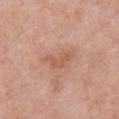The lesion was photographed on a routine skin check and not biopsied; there is no pathology result.
Cropped from a total-body skin-imaging series; the visible field is about 15 mm.
Longest diameter approximately 4.5 mm.
This is a white-light tile.
A female subject aged around 60.
Located on the front of the torso.